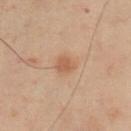biopsy status: total-body-photography surveillance lesion; no biopsy
site: the chest
image: ~15 mm crop, total-body skin-cancer survey
patient: male, in their mid-50s
lesion size: ~2.5 mm (longest diameter)
lighting: cross-polarized illumination